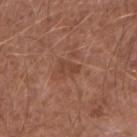Q: Was a biopsy performed?
A: total-body-photography surveillance lesion; no biopsy
Q: Automated lesion metrics?
A: an area of roughly 3.5 mm², an outline eccentricity of about 0.85 (0 = round, 1 = elongated), and a shape-asymmetry score of about 0.35 (0 = symmetric); an average lesion color of about L≈43 a*≈23 b*≈28 (CIELAB), roughly 7 lightness units darker than nearby skin, and a normalized border contrast of about 5.5; internal color variation of about 1.5 on a 0–10 scale and radial color variation of about 0.5
Q: How was the tile lit?
A: white-light
Q: What is the anatomic site?
A: the arm
Q: Lesion size?
A: ≈2.5 mm
Q: Patient demographics?
A: male, aged 63–67
Q: How was this image acquired?
A: 15 mm crop, total-body photography